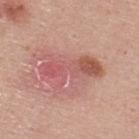Assessment:
Recorded during total-body skin imaging; not selected for excision or biopsy.
Clinical summary:
This image is a 15 mm lesion crop taken from a total-body photograph. The subject is a male aged approximately 25. The lesion is on the upper back. The tile uses white-light illumination. The total-body-photography lesion software estimated a footprint of about 15 mm², an outline eccentricity of about 0.9 (0 = round, 1 = elongated), and two-axis asymmetry of about 0.35. And it measured a lesion color around L≈56 a*≈28 b*≈25 in CIELAB, about 10 CIELAB-L* units darker than the surrounding skin, and a lesion-to-skin contrast of about 6.5 (normalized; higher = more distinct). The analysis additionally found a border-irregularity index near 7/10, a color-variation rating of about 8/10, and radial color variation of about 2.5.